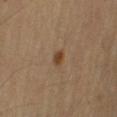Clinical summary: This is a cross-polarized tile. A lesion tile, about 15 mm wide, cut from a 3D total-body photograph. The total-body-photography lesion software estimated an average lesion color of about L≈37 a*≈16 b*≈29 (CIELAB). It also reported internal color variation of about 1.5 on a 0–10 scale. And it measured a detector confidence of about 100 out of 100 that the crop contains a lesion. The lesion is on the right upper arm. A male subject aged around 60.